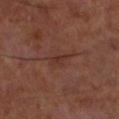Captured during whole-body skin photography for melanoma surveillance; the lesion was not biopsied. A male subject, aged around 70. The lesion is located on the left lower leg. Cropped from a whole-body photographic skin survey; the tile spans about 15 mm.